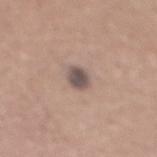Captured during whole-body skin photography for melanoma surveillance; the lesion was not biopsied. A male subject aged 43–47. Measured at roughly 2.5 mm in maximum diameter. Imaged with white-light lighting. The lesion is located on the mid back. A 15 mm crop from a total-body photograph taken for skin-cancer surveillance.